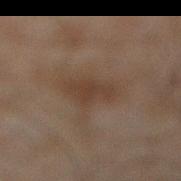workup: no biopsy performed (imaged during a skin exam); lighting: cross-polarized; subject: male, aged 43 to 47; automated lesion analysis: border irregularity of about 2.5 on a 0–10 scale; size: about 4 mm; location: the leg; image: ~15 mm tile from a whole-body skin photo.